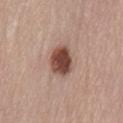{"biopsy_status": "not biopsied; imaged during a skin examination", "lesion_size": {"long_diameter_mm_approx": 3.5}, "image": {"source": "total-body photography crop", "field_of_view_mm": 15}, "patient": {"sex": "female", "age_approx": 75}, "lighting": "white-light", "automated_metrics": {"area_mm2_approx": 9.0, "eccentricity": 0.65, "border_irregularity_0_10": 1.5, "color_variation_0_10": 4.5, "nevus_likeness_0_100": 100}, "site": "lower back"}